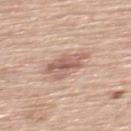Impression:
The lesion was photographed on a routine skin check and not biopsied; there is no pathology result.
Acquisition and patient details:
The subject is a male aged 58–62. Approximately 4.5 mm at its widest. Imaged with white-light lighting. Automated tile analysis of the lesion measured a footprint of about 9.5 mm², a shape eccentricity near 0.75, and two-axis asymmetry of about 0.3. The analysis additionally found a lesion–skin lightness drop of about 11 and a normalized lesion–skin contrast near 7. It also reported a border-irregularity index near 3.5/10 and a within-lesion color-variation index near 5.5/10. It also reported an automated nevus-likeness rating near 0 out of 100 and a lesion-detection confidence of about 100/100. Cropped from a whole-body photographic skin survey; the tile spans about 15 mm. The lesion is on the upper back.